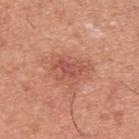Impression: Captured during whole-body skin photography for melanoma surveillance; the lesion was not biopsied. Acquisition and patient details: The lesion's longest dimension is about 5 mm. This image is a 15 mm lesion crop taken from a total-body photograph. This is a white-light tile. Located on the upper back. A male patient in their mid- to late 40s.